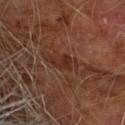Q: Was this lesion biopsied?
A: catalogued during a skin exam; not biopsied
Q: What is the imaging modality?
A: 15 mm crop, total-body photography
Q: What are the patient's age and sex?
A: male, in their 60s
Q: What is the anatomic site?
A: the chest
Q: Automated lesion metrics?
A: an average lesion color of about L≈28 a*≈21 b*≈26 (CIELAB), roughly 6 lightness units darker than nearby skin, and a lesion-to-skin contrast of about 7 (normalized; higher = more distinct); lesion-presence confidence of about 95/100
Q: Lesion size?
A: ~3 mm (longest diameter)
Q: What lighting was used for the tile?
A: cross-polarized